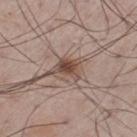<lesion>
<biopsy_status>not biopsied; imaged during a skin examination</biopsy_status>
<lighting>white-light</lighting>
<lesion_size>
  <long_diameter_mm_approx>3.0</long_diameter_mm_approx>
</lesion_size>
<image>
  <source>total-body photography crop</source>
  <field_of_view_mm>15</field_of_view_mm>
</image>
<patient>
  <sex>male</sex>
  <age_approx>60</age_approx>
</patient>
<site>left thigh</site>
</lesion>